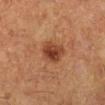Imaged during a routine full-body skin examination; the lesion was not biopsied and no histopathology is available.
The tile uses cross-polarized illumination.
Located on the left lower leg.
Cropped from a whole-body photographic skin survey; the tile spans about 15 mm.
Measured at roughly 3.5 mm in maximum diameter.
A female subject aged 48–52.